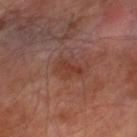Findings:
• workup · catalogued during a skin exam; not biopsied
• size · ~3 mm (longest diameter)
• subject · male, about 70 years old
• site · the right lower leg
• imaging modality · ~15 mm crop, total-body skin-cancer survey